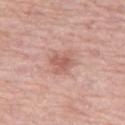Imaged during a routine full-body skin examination; the lesion was not biopsied and no histopathology is available. The tile uses white-light illumination. Automated image analysis of the tile measured a shape eccentricity near 0.4 and two-axis asymmetry of about 0.2. The analysis additionally found an average lesion color of about L≈60 a*≈23 b*≈27 (CIELAB) and a lesion-to-skin contrast of about 6 (normalized; higher = more distinct). The subject is a female roughly 70 years of age. On the right thigh. A region of skin cropped from a whole-body photographic capture, roughly 15 mm wide. The lesion's longest dimension is about 3.5 mm.